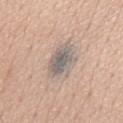No biopsy was performed on this lesion — it was imaged during a full skin examination and was not determined to be concerning. Automated image analysis of the tile measured an eccentricity of roughly 0.8 and a symmetry-axis asymmetry near 0.25. The analysis additionally found a border-irregularity index near 3/10, a within-lesion color-variation index near 4.5/10, and peripheral color asymmetry of about 1.5. The tile uses white-light illumination. A male patient in their mid-50s. The recorded lesion diameter is about 5 mm. Cropped from a whole-body photographic skin survey; the tile spans about 15 mm.